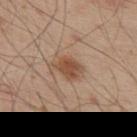Part of a total-body skin-imaging series; this lesion was reviewed on a skin check and was not flagged for biopsy.
The lesion is on the upper back.
A male subject about 55 years old.
Cropped from a total-body skin-imaging series; the visible field is about 15 mm.